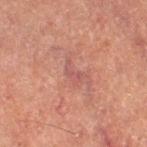biopsy status: total-body-photography surveillance lesion; no biopsy | site: the right thigh | size: ~2.5 mm (longest diameter) | image-analysis metrics: a footprint of about 2.5 mm², an outline eccentricity of about 0.85 (0 = round, 1 = elongated), and a shape-asymmetry score of about 0.65 (0 = symmetric); a lesion–skin lightness drop of about 5 and a normalized lesion–skin contrast near 5 | image: ~15 mm crop, total-body skin-cancer survey | subject: female, in their 60s.